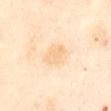notes: imaged on a skin check; not biopsied
imaging modality: ~15 mm tile from a whole-body skin photo
patient: female, approximately 55 years of age
body site: the abdomen